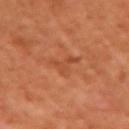Imaged during a routine full-body skin examination; the lesion was not biopsied and no histopathology is available.
The lesion's longest dimension is about 4.5 mm.
The patient is a female aged around 50.
From the left upper arm.
A 15 mm close-up tile from a total-body photography series done for melanoma screening.